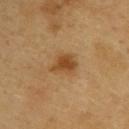Findings:
* biopsy status: total-body-photography surveillance lesion; no biopsy
* patient: male, in their 60s
* tile lighting: cross-polarized illumination
* body site: the upper back
* acquisition: ~15 mm crop, total-body skin-cancer survey
* image-analysis metrics: an automated nevus-likeness rating near 90 out of 100